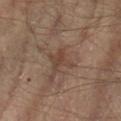Recorded during total-body skin imaging; not selected for excision or biopsy. A male subject in their mid- to late 60s. The lesion is on the left forearm. A roughly 15 mm field-of-view crop from a total-body skin photograph.